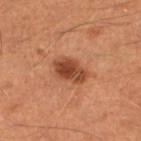patient=male, aged 38 to 42 | location=the left lower leg | diameter=about 4 mm | lighting=cross-polarized | acquisition=~15 mm crop, total-body skin-cancer survey.